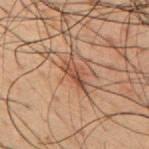Notes:
• biopsy status — no biopsy performed (imaged during a skin exam)
• diameter — ~3 mm (longest diameter)
• lighting — cross-polarized illumination
• subject — male, aged approximately 50
• site — the mid back
• imaging modality — total-body-photography crop, ~15 mm field of view